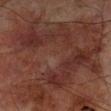Clinical impression:
Imaged during a routine full-body skin examination; the lesion was not biopsied and no histopathology is available.
Background:
On the right lower leg. A male patient about 70 years old. Approximately 15.5 mm at its widest. A 15 mm close-up tile from a total-body photography series done for melanoma screening.